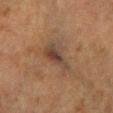Assessment:
Part of a total-body skin-imaging series; this lesion was reviewed on a skin check and was not flagged for biopsy.
Clinical summary:
On the right lower leg. An algorithmic analysis of the crop reported an area of roughly 10 mm² and a symmetry-axis asymmetry near 0.4. It also reported a border-irregularity index near 5/10, a color-variation rating of about 7/10, and a peripheral color-asymmetry measure near 2. Longest diameter approximately 5 mm. This is a cross-polarized tile. Cropped from a total-body skin-imaging series; the visible field is about 15 mm. A male subject, aged around 70.